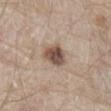Assessment: Imaged during a routine full-body skin examination; the lesion was not biopsied and no histopathology is available. Acquisition and patient details: This is a white-light tile. Automated image analysis of the tile measured an area of roughly 6.5 mm², a shape eccentricity near 0.45, and two-axis asymmetry of about 0.3. The analysis additionally found border irregularity of about 2.5 on a 0–10 scale, internal color variation of about 4.5 on a 0–10 scale, and radial color variation of about 1.5. The software also gave an automated nevus-likeness rating near 80 out of 100 and a lesion-detection confidence of about 100/100. A 15 mm crop from a total-body photograph taken for skin-cancer surveillance. The patient is a male in their 80s. Measured at roughly 3 mm in maximum diameter. The lesion is on the front of the torso.